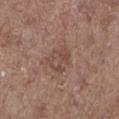Imaged during a routine full-body skin examination; the lesion was not biopsied and no histopathology is available. The total-body-photography lesion software estimated a mean CIELAB color near L≈47 a*≈19 b*≈24, roughly 6 lightness units darker than nearby skin, and a normalized border contrast of about 5. The software also gave border irregularity of about 3.5 on a 0–10 scale, a color-variation rating of about 4.5/10, and peripheral color asymmetry of about 1.5. Approximately 3 mm at its widest. On the left lower leg. A male subject, aged around 60. A 15 mm crop from a total-body photograph taken for skin-cancer surveillance.